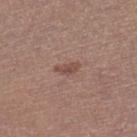No biopsy was performed on this lesion — it was imaged during a full skin examination and was not determined to be concerning. Captured under white-light illumination. A female subject aged around 25. About 2.5 mm across. From the right lower leg. A lesion tile, about 15 mm wide, cut from a 3D total-body photograph.